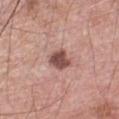Image and clinical context: A male patient, approximately 55 years of age. A 15 mm close-up tile from a total-body photography series done for melanoma screening. Automated tile analysis of the lesion measured a lesion area of about 6.5 mm² and a shape eccentricity near 0.4. And it measured an average lesion color of about L≈50 a*≈22 b*≈23 (CIELAB) and a lesion-to-skin contrast of about 10 (normalized; higher = more distinct). The analysis additionally found border irregularity of about 2.5 on a 0–10 scale, internal color variation of about 3.5 on a 0–10 scale, and peripheral color asymmetry of about 1. The analysis additionally found a nevus-likeness score of about 55/100 and a detector confidence of about 100 out of 100 that the crop contains a lesion. The tile uses white-light illumination. The lesion is on the chest. About 3 mm across.